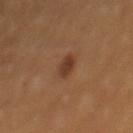No biopsy was performed on this lesion — it was imaged during a full skin examination and was not determined to be concerning.
A 15 mm crop from a total-body photograph taken for skin-cancer surveillance.
About 3.5 mm across.
This is a cross-polarized tile.
A male subject in their mid- to late 50s.
The lesion is on the back.